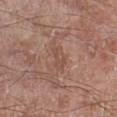No biopsy was performed on this lesion — it was imaged during a full skin examination and was not determined to be concerning.
A male patient in their 60s.
Located on the leg.
Cropped from a whole-body photographic skin survey; the tile spans about 15 mm.